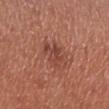| field | value |
|---|---|
| workup | no biopsy performed (imaged during a skin exam) |
| lesion diameter | ~3 mm (longest diameter) |
| image source | ~15 mm crop, total-body skin-cancer survey |
| patient | male, aged around 70 |
| body site | the left lower leg |